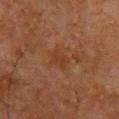On the chest.
This is a cross-polarized tile.
A male patient in their mid-60s.
Approximately 3 mm at its widest.
Cropped from a whole-body photographic skin survey; the tile spans about 15 mm.